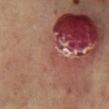Q: Was this lesion biopsied?
A: catalogued during a skin exam; not biopsied
Q: Automated lesion metrics?
A: border irregularity of about 3 on a 0–10 scale and peripheral color asymmetry of about 0
Q: Lesion size?
A: ≈1 mm
Q: Lesion location?
A: the chest
Q: What kind of image is this?
A: ~15 mm tile from a whole-body skin photo
Q: Who is the patient?
A: male, aged 63–67